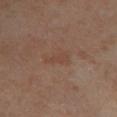Captured during whole-body skin photography for melanoma surveillance; the lesion was not biopsied.
A region of skin cropped from a whole-body photographic capture, roughly 15 mm wide.
The lesion is located on the right lower leg.
A female subject, in their mid- to late 50s.
The lesion's longest dimension is about 3 mm.